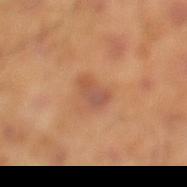  biopsy_status: not biopsied; imaged during a skin examination
  patient:
    sex: male
    age_approx: 45
  automated_metrics:
    nevus_likeness_0_100: 0
    lesion_detection_confidence_0_100: 100
  image:
    source: total-body photography crop
    field_of_view_mm: 15
  site: left lower leg